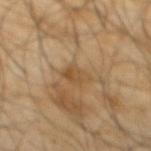biopsy status: imaged on a skin check; not biopsied
TBP lesion metrics: an area of roughly 5 mm², a shape eccentricity near 0.5, and two-axis asymmetry of about 0.2; internal color variation of about 3.5 on a 0–10 scale and a peripheral color-asymmetry measure near 1; a classifier nevus-likeness of about 0/100 and lesion-presence confidence of about 95/100
illumination: cross-polarized illumination
site: the back
image: 15 mm crop, total-body photography
subject: male, roughly 65 years of age